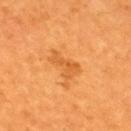Findings:
• biopsy status · no biopsy performed (imaged during a skin exam)
• acquisition · ~15 mm tile from a whole-body skin photo
• location · the back
• patient · male, aged around 60